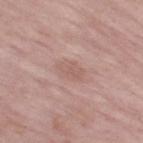Recorded during total-body skin imaging; not selected for excision or biopsy. The lesion's longest dimension is about 3 mm. A 15 mm close-up tile from a total-body photography series done for melanoma screening. A female patient, in their 70s. The tile uses white-light illumination. The total-body-photography lesion software estimated border irregularity of about 2.5 on a 0–10 scale, a color-variation rating of about 1.5/10, and peripheral color asymmetry of about 0.5. The software also gave a classifier nevus-likeness of about 0/100 and a lesion-detection confidence of about 100/100. On the right thigh.